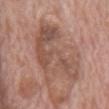<lesion>
<biopsy_status>not biopsied; imaged during a skin examination</biopsy_status>
<patient>
  <sex>male</sex>
  <age_approx>70</age_approx>
</patient>
<lighting>white-light</lighting>
<automated_metrics>
  <area_mm2_approx>32.0</area_mm2_approx>
  <eccentricity>0.8</eccentricity>
  <shape_asymmetry>0.55</shape_asymmetry>
  <nevus_likeness_0_100>0</nevus_likeness_0_100>
  <lesion_detection_confidence_0_100>100</lesion_detection_confidence_0_100>
</automated_metrics>
<site>mid back</site>
<image>
  <source>total-body photography crop</source>
  <field_of_view_mm>15</field_of_view_mm>
</image>
</lesion>